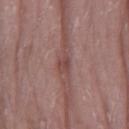biopsy_status: not biopsied; imaged during a skin examination
patient:
  sex: female
  age_approx: 70
lighting: white-light
automated_metrics:
  area_mm2_approx: 3.5
  eccentricity: 0.85
  shape_asymmetry: 0.4
  cielab_L: 45
  cielab_a: 21
  cielab_b: 22
  vs_skin_darker_L: 9.0
  vs_skin_contrast_norm: 7.0
  nevus_likeness_0_100: 0
  lesion_detection_confidence_0_100: 95
site: leg
image:
  source: total-body photography crop
  field_of_view_mm: 15
lesion_size:
  long_diameter_mm_approx: 2.5